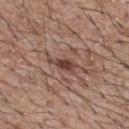This lesion was catalogued during total-body skin photography and was not selected for biopsy. A male patient, roughly 65 years of age. Located on the mid back. The total-body-photography lesion software estimated an automated nevus-likeness rating near 25 out of 100 and a lesion-detection confidence of about 100/100. Imaged with white-light lighting. A roughly 15 mm field-of-view crop from a total-body skin photograph.